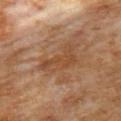The lesion was tiled from a total-body skin photograph and was not biopsied. Cropped from a whole-body photographic skin survey; the tile spans about 15 mm. A female patient, aged around 50. Captured under cross-polarized illumination. Measured at roughly 5 mm in maximum diameter. Located on the left upper arm.